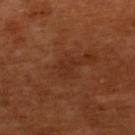Recorded during total-body skin imaging; not selected for excision or biopsy.
The patient is a female aged around 55.
A close-up tile cropped from a whole-body skin photograph, about 15 mm across.
The lesion is on the upper back.
Captured under cross-polarized illumination.